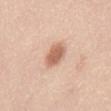* workup · catalogued during a skin exam; not biopsied
* illumination · white-light illumination
* acquisition · 15 mm crop, total-body photography
* subject · male, aged approximately 45
* automated metrics · a lesion area of about 6.5 mm² and a shape eccentricity near 0.75; a lesion-to-skin contrast of about 8 (normalized; higher = more distinct); border irregularity of about 2 on a 0–10 scale, a within-lesion color-variation index near 2/10, and a peripheral color-asymmetry measure near 0.5; an automated nevus-likeness rating near 95 out of 100 and a lesion-detection confidence of about 100/100
* diameter · about 3.5 mm
* anatomic site · the abdomen